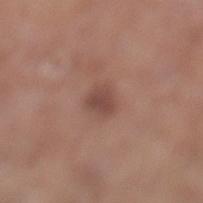notes: no biopsy performed (imaged during a skin exam)
image source: ~15 mm tile from a whole-body skin photo
TBP lesion metrics: a lesion color around L≈46 a*≈21 b*≈24 in CIELAB, a lesion–skin lightness drop of about 9, and a normalized lesion–skin contrast near 7; a border-irregularity rating of about 2.5/10, internal color variation of about 2 on a 0–10 scale, and radial color variation of about 0.5; a nevus-likeness score of about 50/100 and a lesion-detection confidence of about 100/100
illumination: white-light
patient: female, roughly 75 years of age
lesion size: about 2.5 mm
body site: the left lower leg